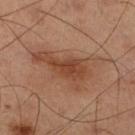notes: catalogued during a skin exam; not biopsied
tile lighting: cross-polarized
TBP lesion metrics: a shape eccentricity near 0.85; a lesion color around L≈44 a*≈21 b*≈31 in CIELAB, roughly 9 lightness units darker than nearby skin, and a normalized border contrast of about 7
location: the right lower leg
image: ~15 mm crop, total-body skin-cancer survey
diameter: ≈7.5 mm
subject: male, aged around 60